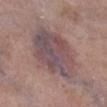This lesion was catalogued during total-body skin photography and was not selected for biopsy. A male patient, about 75 years old. About 7 mm across. A 15 mm close-up extracted from a 3D total-body photography capture. Captured under white-light illumination. The lesion is on the right lower leg. The lesion-visualizer software estimated a shape eccentricity near 0.6 and two-axis asymmetry of about 0.15. The software also gave a lesion color around L≈48 a*≈17 b*≈15 in CIELAB, a lesion–skin lightness drop of about 10, and a normalized border contrast of about 8.5. The analysis additionally found an automated nevus-likeness rating near 0 out of 100 and a lesion-detection confidence of about 75/100.